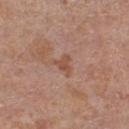| feature | finding |
|---|---|
| tile lighting | white-light |
| image source | 15 mm crop, total-body photography |
| automated metrics | an eccentricity of roughly 0.55 and a shape-asymmetry score of about 0.6 (0 = symmetric); a border-irregularity rating of about 6/10 and a color-variation rating of about 1.5/10; a classifier nevus-likeness of about 0/100 and a lesion-detection confidence of about 100/100 |
| subject | female, aged 53 to 57 |
| body site | the right lower leg |